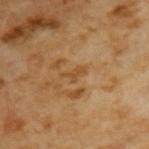Clinical impression:
Captured during whole-body skin photography for melanoma surveillance; the lesion was not biopsied.
Image and clinical context:
A 15 mm close-up tile from a total-body photography series done for melanoma screening. The patient is a male aged 63–67.